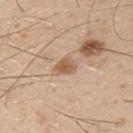Findings:
* biopsy status — total-body-photography surveillance lesion; no biopsy
* image source — total-body-photography crop, ~15 mm field of view
* automated metrics — an outline eccentricity of about 0.7 (0 = round, 1 = elongated); an average lesion color of about L≈58 a*≈19 b*≈32 (CIELAB), a lesion–skin lightness drop of about 11, and a lesion-to-skin contrast of about 7.5 (normalized; higher = more distinct)
* subject — male, aged around 30
* body site — the left upper arm
* illumination — white-light